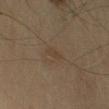Automated image analysis of the tile measured a detector confidence of about 100 out of 100 that the crop contains a lesion. Located on the left upper arm. Captured under cross-polarized illumination. The subject is a male aged 53–57. The lesion's longest dimension is about 2.5 mm. A lesion tile, about 15 mm wide, cut from a 3D total-body photograph.